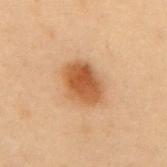Q: Automated lesion metrics?
A: a symmetry-axis asymmetry near 0.15; a border-irregularity index near 1.5/10, internal color variation of about 4 on a 0–10 scale, and a peripheral color-asymmetry measure near 1; a nevus-likeness score of about 100/100
Q: What is the imaging modality?
A: ~15 mm tile from a whole-body skin photo
Q: Illumination type?
A: cross-polarized illumination
Q: What is the lesion's diameter?
A: about 4.5 mm
Q: What are the patient's age and sex?
A: male, roughly 55 years of age
Q: Where on the body is the lesion?
A: the mid back